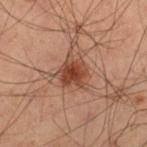| feature | finding |
|---|---|
| workup | catalogued during a skin exam; not biopsied |
| site | the left thigh |
| patient | male, approximately 60 years of age |
| diameter | about 4 mm |
| imaging modality | ~15 mm tile from a whole-body skin photo |
| lighting | cross-polarized illumination |